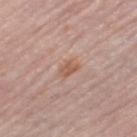biopsy status — imaged on a skin check; not biopsied | image — ~15 mm tile from a whole-body skin photo | body site — the leg | tile lighting — white-light illumination | automated metrics — a lesion color around L≈57 a*≈21 b*≈29 in CIELAB, about 8 CIELAB-L* units darker than the surrounding skin, and a normalized border contrast of about 6.5; border irregularity of about 2.5 on a 0–10 scale, a color-variation rating of about 2/10, and radial color variation of about 1; an automated nevus-likeness rating near 25 out of 100 | patient — female, aged 63–67 | diameter — about 2.5 mm.